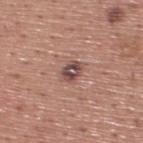Recorded during total-body skin imaging; not selected for excision or biopsy.
Located on the upper back.
Captured under white-light illumination.
A 15 mm close-up extracted from a 3D total-body photography capture.
A male subject aged approximately 45.
About 2.5 mm across.